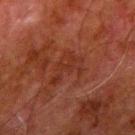The total-body-photography lesion software estimated a lesion–skin lightness drop of about 4 and a lesion-to-skin contrast of about 5 (normalized; higher = more distinct). And it measured a classifier nevus-likeness of about 0/100 and a detector confidence of about 100 out of 100 that the crop contains a lesion. A 15 mm close-up tile from a total-body photography series done for melanoma screening. A male subject, in their 80s. The tile uses cross-polarized illumination. The lesion is located on the right upper arm. About 4 mm across.